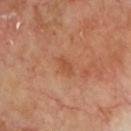Clinical impression: Captured during whole-body skin photography for melanoma surveillance; the lesion was not biopsied. Background: Approximately 2.5 mm at its widest. The lesion is located on the chest. A male subject, aged 68 to 72. Cropped from a total-body skin-imaging series; the visible field is about 15 mm. The tile uses cross-polarized illumination.